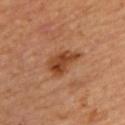{"biopsy_status": "not biopsied; imaged during a skin examination", "automated_metrics": {"shape_asymmetry": 0.3}, "image": {"source": "total-body photography crop", "field_of_view_mm": 15}, "lesion_size": {"long_diameter_mm_approx": 4.0}, "site": "upper back", "lighting": "cross-polarized", "patient": {"sex": "female", "age_approx": 70}}